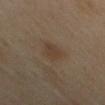| key | value |
|---|---|
| notes | imaged on a skin check; not biopsied |
| patient | female, aged 58–62 |
| tile lighting | cross-polarized illumination |
| imaging modality | ~15 mm tile from a whole-body skin photo |
| site | the leg |